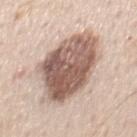Q: Patient demographics?
A: male, approximately 60 years of age
Q: Lesion location?
A: the mid back
Q: What lighting was used for the tile?
A: white-light illumination
Q: How large is the lesion?
A: ~9 mm (longest diameter)
Q: What is the imaging modality?
A: 15 mm crop, total-body photography
Q: Automated lesion metrics?
A: internal color variation of about 7 on a 0–10 scale and radial color variation of about 2.5